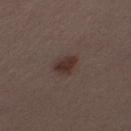Context:
The total-body-photography lesion software estimated a footprint of about 5.5 mm², an eccentricity of roughly 0.65, and two-axis asymmetry of about 0.3. A female subject in their 30s. Measured at roughly 3 mm in maximum diameter. A 15 mm close-up extracted from a 3D total-body photography capture. Located on the left thigh. This is a white-light tile.